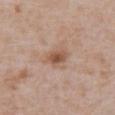The lesion's longest dimension is about 2.5 mm.
Captured under white-light illumination.
The lesion is on the abdomen.
A 15 mm close-up extracted from a 3D total-body photography capture.
The lesion-visualizer software estimated internal color variation of about 3.5 on a 0–10 scale and peripheral color asymmetry of about 1.
The subject is a male aged approximately 65.